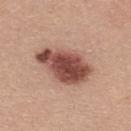notes = no biopsy performed (imaged during a skin exam)
patient = female, in their mid- to late 30s
image-analysis metrics = a lesion color around L≈49 a*≈24 b*≈26 in CIELAB, about 18 CIELAB-L* units darker than the surrounding skin, and a normalized border contrast of about 12; a classifier nevus-likeness of about 90/100 and a lesion-detection confidence of about 100/100
imaging modality = ~15 mm crop, total-body skin-cancer survey
lesion size = ≈6.5 mm
lighting = white-light illumination
site = the upper back